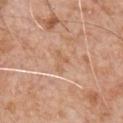Part of a total-body skin-imaging series; this lesion was reviewed on a skin check and was not flagged for biopsy. On the chest. A male subject, aged 63 to 67. Cropped from a whole-body photographic skin survey; the tile spans about 15 mm.